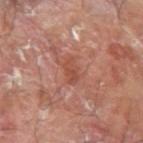lighting: cross-polarized illumination | patient: male, about 65 years old | lesion diameter: about 3 mm | image source: 15 mm crop, total-body photography | location: the left forearm.